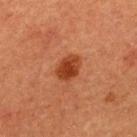Impression: Captured during whole-body skin photography for melanoma surveillance; the lesion was not biopsied. Image and clinical context: On the upper back. A male subject, about 50 years old. The total-body-photography lesion software estimated a border-irregularity rating of about 1.5/10, internal color variation of about 3 on a 0–10 scale, and radial color variation of about 1. The analysis additionally found a nevus-likeness score of about 100/100. Longest diameter approximately 3 mm. Captured under cross-polarized illumination. A 15 mm crop from a total-body photograph taken for skin-cancer surveillance.